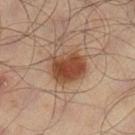The lesion was tiled from a total-body skin photograph and was not biopsied. Captured under cross-polarized illumination. The recorded lesion diameter is about 4.5 mm. Automated tile analysis of the lesion measured a footprint of about 14 mm², an outline eccentricity of about 0.55 (0 = round, 1 = elongated), and a shape-asymmetry score of about 0.15 (0 = symmetric). The analysis additionally found an average lesion color of about L≈43 a*≈20 b*≈30 (CIELAB). Cropped from a total-body skin-imaging series; the visible field is about 15 mm. A male patient, aged approximately 65. On the left lower leg.